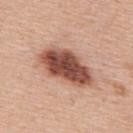<lesion>
  <biopsy_status>not biopsied; imaged during a skin examination</biopsy_status>
  <lesion_size>
    <long_diameter_mm_approx>7.0</long_diameter_mm_approx>
  </lesion_size>
  <automated_metrics>
    <area_mm2_approx>19.0</area_mm2_approx>
    <eccentricity>0.85</eccentricity>
    <shape_asymmetry>0.2</shape_asymmetry>
    <nevus_likeness_0_100>85</nevus_likeness_0_100>
    <lesion_detection_confidence_0_100>100</lesion_detection_confidence_0_100>
  </automated_metrics>
  <site>upper back</site>
  <image>
    <source>total-body photography crop</source>
    <field_of_view_mm>15</field_of_view_mm>
  </image>
  <lighting>white-light</lighting>
  <patient>
    <sex>male</sex>
    <age_approx>60</age_approx>
  </patient>
</lesion>